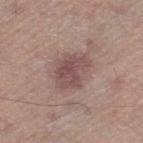Part of a total-body skin-imaging series; this lesion was reviewed on a skin check and was not flagged for biopsy. A roughly 15 mm field-of-view crop from a total-body skin photograph. Located on the leg. A male subject about 65 years old. Automated tile analysis of the lesion measured an outline eccentricity of about 0.65 (0 = round, 1 = elongated) and two-axis asymmetry of about 0.2. The analysis additionally found a nevus-likeness score of about 20/100 and a detector confidence of about 100 out of 100 that the crop contains a lesion.